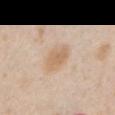{
  "biopsy_status": "not biopsied; imaged during a skin examination",
  "patient": {
    "sex": "male",
    "age_approx": 35
  },
  "site": "left upper arm",
  "image": {
    "source": "total-body photography crop",
    "field_of_view_mm": 15
  },
  "automated_metrics": {
    "border_irregularity_0_10": 2.0,
    "color_variation_0_10": 2.5,
    "peripheral_color_asymmetry": 1.0
  },
  "lesion_size": {
    "long_diameter_mm_approx": 3.5
  }
}